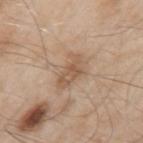biopsy status: no biopsy performed (imaged during a skin exam) | size: ~4 mm (longest diameter) | automated metrics: internal color variation of about 3.5 on a 0–10 scale and a peripheral color-asymmetry measure near 1 | tile lighting: white-light illumination | acquisition: total-body-photography crop, ~15 mm field of view | site: the left upper arm | patient: male, aged approximately 55.